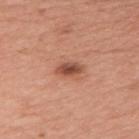biopsy status: imaged on a skin check; not biopsied | acquisition: total-body-photography crop, ~15 mm field of view | TBP lesion metrics: a border-irregularity index near 2/10 and a peripheral color-asymmetry measure near 1.5; a nevus-likeness score of about 90/100 | site: the left upper arm | lighting: white-light illumination | diameter: ~3 mm (longest diameter) | subject: female, about 40 years old.